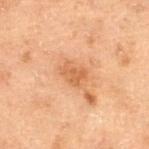Imaged during a routine full-body skin examination; the lesion was not biopsied and no histopathology is available. The patient is a male about 70 years old. Automated tile analysis of the lesion measured an area of roughly 4.5 mm², an eccentricity of roughly 0.6, and two-axis asymmetry of about 0.3. The analysis additionally found border irregularity of about 3 on a 0–10 scale, internal color variation of about 1.5 on a 0–10 scale, and a peripheral color-asymmetry measure near 0.5. And it measured a nevus-likeness score of about 15/100 and a detector confidence of about 100 out of 100 that the crop contains a lesion. This is a cross-polarized tile. On the left thigh. Cropped from a whole-body photographic skin survey; the tile spans about 15 mm.